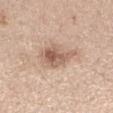biopsy status: catalogued during a skin exam; not biopsied | tile lighting: white-light illumination | image source: 15 mm crop, total-body photography | patient: female, aged around 45 | anatomic site: the right forearm.